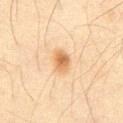A roughly 15 mm field-of-view crop from a total-body skin photograph.
Located on the abdomen.
The recorded lesion diameter is about 3 mm.
A male subject aged approximately 65.
An algorithmic analysis of the crop reported an eccentricity of roughly 0.75 and a symmetry-axis asymmetry near 0.2. The software also gave a classifier nevus-likeness of about 95/100 and a lesion-detection confidence of about 100/100.